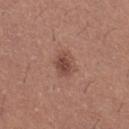workup = imaged on a skin check; not biopsied | lesion diameter = ≈3 mm | automated lesion analysis = a lesion area of about 5.5 mm² and a shape eccentricity near 0.6; a lesion color around L≈47 a*≈22 b*≈26 in CIELAB and roughly 10 lightness units darker than nearby skin; a border-irregularity rating of about 2/10 and radial color variation of about 1 | imaging modality = 15 mm crop, total-body photography | body site = the right thigh | patient = female, approximately 25 years of age | lighting = white-light.